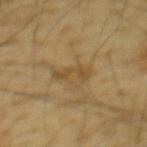Q: Was this lesion biopsied?
A: total-body-photography surveillance lesion; no biopsy
Q: What is the lesion's diameter?
A: ~3.5 mm (longest diameter)
Q: What is the imaging modality?
A: ~15 mm tile from a whole-body skin photo
Q: Where on the body is the lesion?
A: the mid back
Q: Automated lesion metrics?
A: a normalized lesion–skin contrast near 6
Q: Who is the patient?
A: male, aged around 65
Q: What lighting was used for the tile?
A: cross-polarized